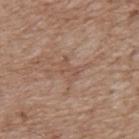Clinical impression:
This lesion was catalogued during total-body skin photography and was not selected for biopsy.
Background:
On the upper back. Approximately 3 mm at its widest. A male subject approximately 70 years of age. Automated image analysis of the tile measured a lesion area of about 4 mm² and a symmetry-axis asymmetry near 0.35. It also reported a lesion color around L≈52 a*≈18 b*≈28 in CIELAB. And it measured border irregularity of about 5.5 on a 0–10 scale, a within-lesion color-variation index near 0/10, and radial color variation of about 0. And it measured a classifier nevus-likeness of about 0/100 and a lesion-detection confidence of about 85/100. The tile uses white-light illumination. A region of skin cropped from a whole-body photographic capture, roughly 15 mm wide.